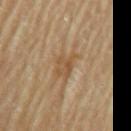Notes:
– workup · total-body-photography surveillance lesion; no biopsy
– lesion diameter · ~4 mm (longest diameter)
– image · ~15 mm tile from a whole-body skin photo
– body site · the left upper arm
– image-analysis metrics · a lesion area of about 6.5 mm², a shape eccentricity near 0.8, and two-axis asymmetry of about 0.3; an automated nevus-likeness rating near 0 out of 100 and a detector confidence of about 80 out of 100 that the crop contains a lesion
– tile lighting · cross-polarized
– patient · male, aged 83 or over